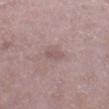| feature | finding |
|---|---|
| notes | catalogued during a skin exam; not biopsied |
| lesion diameter | ~2.5 mm (longest diameter) |
| image | total-body-photography crop, ~15 mm field of view |
| lighting | white-light illumination |
| patient | male, aged around 70 |
| anatomic site | the right lower leg |